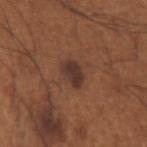No biopsy was performed on this lesion — it was imaged during a full skin examination and was not determined to be concerning. From the left upper arm. Imaged with white-light lighting. A close-up tile cropped from a whole-body skin photograph, about 15 mm across. A male patient approximately 65 years of age. Approximately 3 mm at its widest.